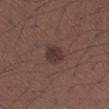Acquisition and patient details: Captured under white-light illumination. Longest diameter approximately 3 mm. The subject is a male roughly 40 years of age. A 15 mm close-up tile from a total-body photography series done for melanoma screening. On the arm.